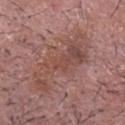Impression: Imaged during a routine full-body skin examination; the lesion was not biopsied and no histopathology is available. Context: Cropped from a total-body skin-imaging series; the visible field is about 15 mm. A male patient roughly 85 years of age or older. The lesion is on the head or neck. Automated image analysis of the tile measured a lesion color around L≈47 a*≈21 b*≈24 in CIELAB. It also reported a border-irregularity rating of about 8/10 and a within-lesion color-variation index near 4.5/10. Approximately 9 mm at its widest.